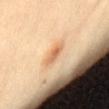{
  "biopsy_status": "not biopsied; imaged during a skin examination",
  "patient": {
    "sex": "female",
    "age_approx": 60
  },
  "site": "lower back",
  "image": {
    "source": "total-body photography crop",
    "field_of_view_mm": 15
  },
  "lesion_size": {
    "long_diameter_mm_approx": 3.0
  },
  "lighting": "cross-polarized"
}